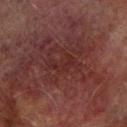Case summary:
* notes: imaged on a skin check; not biopsied
* subject: male, approximately 75 years of age
* site: the right forearm
* lesion diameter: ≈3.5 mm
* image source: ~15 mm crop, total-body skin-cancer survey
* lighting: cross-polarized